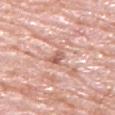follow-up: catalogued during a skin exam; not biopsied
automated metrics: an area of roughly 3 mm² and a shape-asymmetry score of about 0.4 (0 = symmetric); border irregularity of about 4 on a 0–10 scale, a within-lesion color-variation index near 4.5/10, and a peripheral color-asymmetry measure near 1.5; an automated nevus-likeness rating near 0 out of 100 and a detector confidence of about 95 out of 100 that the crop contains a lesion
subject: male, roughly 80 years of age
body site: the right upper arm
tile lighting: white-light
lesion size: ≈2.5 mm
image source: 15 mm crop, total-body photography